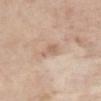A 15 mm crop from a total-body photograph taken for skin-cancer surveillance. The subject is a female approximately 65 years of age. From the abdomen.